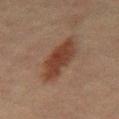workup = total-body-photography surveillance lesion; no biopsy | patient = male, in their mid-60s | size = ≈7 mm | image = ~15 mm crop, total-body skin-cancer survey | site = the mid back.